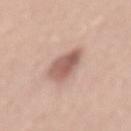Part of a total-body skin-imaging series; this lesion was reviewed on a skin check and was not flagged for biopsy. Longest diameter approximately 5 mm. The patient is a female about 35 years old. The lesion is on the abdomen. Cropped from a total-body skin-imaging series; the visible field is about 15 mm.